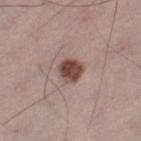  biopsy_status: not biopsied; imaged during a skin examination
  patient:
    sex: male
    age_approx: 40
  lighting: white-light
  automated_metrics:
    area_mm2_approx: 6.5
    eccentricity: 0.35
    cielab_L: 46
    cielab_a: 19
    cielab_b: 22
    vs_skin_contrast_norm: 11.0
    lesion_detection_confidence_0_100: 100
  site: left thigh
  image:
    source: total-body photography crop
    field_of_view_mm: 15
  lesion_size:
    long_diameter_mm_approx: 3.0